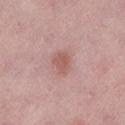Captured during whole-body skin photography for melanoma surveillance; the lesion was not biopsied.
A female subject aged 48 to 52.
The tile uses white-light illumination.
On the leg.
The lesion-visualizer software estimated an area of roughly 4.5 mm², an outline eccentricity of about 0.55 (0 = round, 1 = elongated), and a shape-asymmetry score of about 0.25 (0 = symmetric). It also reported a lesion–skin lightness drop of about 9 and a normalized border contrast of about 6.5. It also reported a border-irregularity rating of about 2/10 and peripheral color asymmetry of about 0.5.
A 15 mm close-up extracted from a 3D total-body photography capture.
Approximately 2.5 mm at its widest.